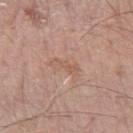Clinical impression:
Imaged during a routine full-body skin examination; the lesion was not biopsied and no histopathology is available.
Background:
Automated tile analysis of the lesion measured a lesion area of about 4 mm² and a shape-asymmetry score of about 0.45 (0 = symmetric). And it measured a border-irregularity index near 5.5/10, a color-variation rating of about 1/10, and radial color variation of about 0.5. It also reported a detector confidence of about 100 out of 100 that the crop contains a lesion. A lesion tile, about 15 mm wide, cut from a 3D total-body photograph. A male patient aged 58–62. Captured under white-light illumination. Located on the left forearm. Approximately 3 mm at its widest.